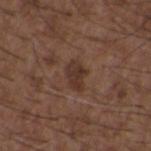  biopsy_status: not biopsied; imaged during a skin examination
  patient:
    sex: male
    age_approx: 50
  site: back
  image:
    source: total-body photography crop
    field_of_view_mm: 15